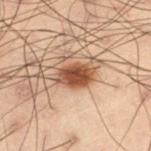This lesion was catalogued during total-body skin photography and was not selected for biopsy.
A male subject in their mid-50s.
The lesion is located on the left thigh.
The tile uses cross-polarized illumination.
A roughly 15 mm field-of-view crop from a total-body skin photograph.
Measured at roughly 4 mm in maximum diameter.